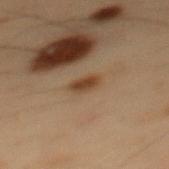follow-up — catalogued during a skin exam; not biopsied
subject — male, about 55 years old
location — the mid back
tile lighting — cross-polarized illumination
lesion diameter — about 2.5 mm
image-analysis metrics — an area of roughly 3.5 mm², an outline eccentricity of about 0.8 (0 = round, 1 = elongated), and a symmetry-axis asymmetry near 0.3; a border-irregularity index near 3/10, internal color variation of about 1.5 on a 0–10 scale, and radial color variation of about 0.5
acquisition — total-body-photography crop, ~15 mm field of view